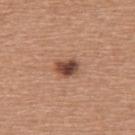No biopsy was performed on this lesion — it was imaged during a full skin examination and was not determined to be concerning.
Imaged with white-light lighting.
Automated tile analysis of the lesion measured an area of roughly 5 mm², an outline eccentricity of about 0.7 (0 = round, 1 = elongated), and a symmetry-axis asymmetry near 0.25. And it measured a lesion color around L≈46 a*≈22 b*≈28 in CIELAB, roughly 15 lightness units darker than nearby skin, and a normalized border contrast of about 11.
A female subject aged 53–57.
The lesion is on the upper back.
Cropped from a whole-body photographic skin survey; the tile spans about 15 mm.